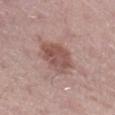image-analysis metrics = a lesion area of about 12 mm² and a shape eccentricity near 0.7; a nevus-likeness score of about 25/100
size = ~5 mm (longest diameter)
tile lighting = white-light
image source = 15 mm crop, total-body photography
anatomic site = the right lower leg
subject = male, aged 48 to 52